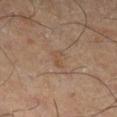Part of a total-body skin-imaging series; this lesion was reviewed on a skin check and was not flagged for biopsy.
The patient is a male approximately 65 years of age.
A 15 mm close-up extracted from a 3D total-body photography capture.
Located on the right lower leg.
Captured under cross-polarized illumination.